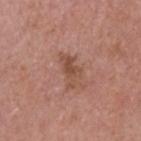No biopsy was performed on this lesion — it was imaged during a full skin examination and was not determined to be concerning. A male patient aged around 75. The recorded lesion diameter is about 4 mm. Captured under white-light illumination. Automated tile analysis of the lesion measured an eccentricity of roughly 0.85 and a shape-asymmetry score of about 0.45 (0 = symmetric). And it measured a lesion–skin lightness drop of about 8 and a normalized lesion–skin contrast near 6.5. The software also gave a border-irregularity rating of about 5/10. It also reported a detector confidence of about 100 out of 100 that the crop contains a lesion. This image is a 15 mm lesion crop taken from a total-body photograph. From the head or neck.